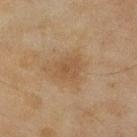This lesion was catalogued during total-body skin photography and was not selected for biopsy. A female subject, roughly 60 years of age. On the leg. This image is a 15 mm lesion crop taken from a total-body photograph. Longest diameter approximately 3 mm. Imaged with cross-polarized lighting.